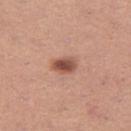Case summary:
– follow-up — total-body-photography surveillance lesion; no biopsy
– anatomic site — the leg
– diameter — about 3 mm
– acquisition — 15 mm crop, total-body photography
– lighting — white-light illumination
– subject — female, in their 30s